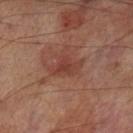The lesion was photographed on a routine skin check and not biopsied; there is no pathology result.